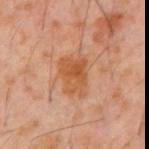Clinical impression:
This lesion was catalogued during total-body skin photography and was not selected for biopsy.
Clinical summary:
Located on the abdomen. The patient is a male about 60 years old. A roughly 15 mm field-of-view crop from a total-body skin photograph. The total-body-photography lesion software estimated a color-variation rating of about 4.5/10 and radial color variation of about 1.5.